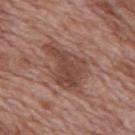  biopsy_status: not biopsied; imaged during a skin examination
  patient:
    sex: male
    age_approx: 70
  image:
    source: total-body photography crop
    field_of_view_mm: 15
  automated_metrics:
    shape_asymmetry: 0.45
    cielab_L: 45
    cielab_a: 21
    cielab_b: 25
    vs_skin_darker_L: 10.0
    border_irregularity_0_10: 5.0
    color_variation_0_10: 3.5
    peripheral_color_asymmetry: 1.0
  lighting: white-light
  site: mid back
  lesion_size:
    long_diameter_mm_approx: 6.5